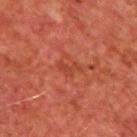workup — catalogued during a skin exam; not biopsied | patient — male, aged 63 to 67 | image source — ~15 mm tile from a whole-body skin photo | diameter — about 2.5 mm | TBP lesion metrics — a footprint of about 2.5 mm², a shape eccentricity near 0.85, and two-axis asymmetry of about 0.55; border irregularity of about 6 on a 0–10 scale and a color-variation rating of about 0/10; an automated nevus-likeness rating near 0 out of 100 and a lesion-detection confidence of about 100/100 | tile lighting — cross-polarized | site — the upper back.